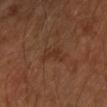- notes: imaged on a skin check; not biopsied
- TBP lesion metrics: an area of roughly 5.5 mm²; a border-irregularity rating of about 5.5/10 and radial color variation of about 0.5; an automated nevus-likeness rating near 0 out of 100 and a detector confidence of about 100 out of 100 that the crop contains a lesion
- site: the left forearm
- acquisition: ~15 mm tile from a whole-body skin photo
- patient: female, about 60 years old